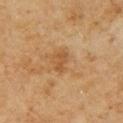Assessment: Captured during whole-body skin photography for melanoma surveillance; the lesion was not biopsied. Context: On the left upper arm. Cropped from a whole-body photographic skin survey; the tile spans about 15 mm. Longest diameter approximately 3 mm. A male subject roughly 60 years of age. Imaged with cross-polarized lighting. The lesion-visualizer software estimated an eccentricity of roughly 0.9 and a shape-asymmetry score of about 0.35 (0 = symmetric).